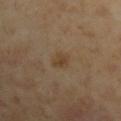<case>
<image>
  <source>total-body photography crop</source>
  <field_of_view_mm>15</field_of_view_mm>
</image>
<lighting>cross-polarized</lighting>
<automated_metrics>
  <eccentricity>0.75</eccentricity>
  <border_irregularity_0_10>2.5</border_irregularity_0_10>
  <color_variation_0_10>2.0</color_variation_0_10>
  <peripheral_color_asymmetry>0.5</peripheral_color_asymmetry>
  <nevus_likeness_0_100>10</nevus_likeness_0_100>
</automated_metrics>
<site>arm</site>
<patient>
  <sex>male</sex>
  <age_approx>45</age_approx>
</patient>
</case>